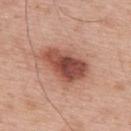Clinical impression:
This lesion was catalogued during total-body skin photography and was not selected for biopsy.
Acquisition and patient details:
An algorithmic analysis of the crop reported a lesion area of about 18 mm² and two-axis asymmetry of about 0.25. The software also gave a lesion color around L≈51 a*≈25 b*≈29 in CIELAB, about 15 CIELAB-L* units darker than the surrounding skin, and a normalized lesion–skin contrast near 10. A roughly 15 mm field-of-view crop from a total-body skin photograph. The subject is a male aged 63–67. From the upper back. Captured under white-light illumination.